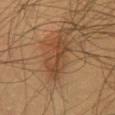This image is a 15 mm lesion crop taken from a total-body photograph. The recorded lesion diameter is about 6 mm. Imaged with cross-polarized lighting. A male patient, roughly 60 years of age. Located on the chest. The total-body-photography lesion software estimated a lesion area of about 10 mm² and a shape eccentricity near 0.95. It also reported a lesion–skin lightness drop of about 9 and a normalized lesion–skin contrast near 7.